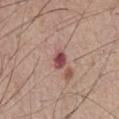Notes:
– follow-up · catalogued during a skin exam; not biopsied
– imaging modality · total-body-photography crop, ~15 mm field of view
– lighting · white-light
– subject · male, aged 53–57
– automated lesion analysis · a lesion color around L≈48 a*≈26 b*≈21 in CIELAB, roughly 14 lightness units darker than nearby skin, and a lesion-to-skin contrast of about 10.5 (normalized; higher = more distinct); a border-irregularity rating of about 2/10, a color-variation rating of about 4/10, and radial color variation of about 1.5; a detector confidence of about 100 out of 100 that the crop contains a lesion
– diameter · about 2.5 mm
– site · the chest